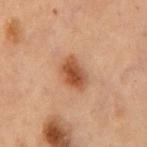Part of a total-body skin-imaging series; this lesion was reviewed on a skin check and was not flagged for biopsy.
An algorithmic analysis of the crop reported a footprint of about 7 mm² and a shape eccentricity near 0.7. And it measured a border-irregularity index near 2/10 and a color-variation rating of about 4/10. And it measured a lesion-detection confidence of about 100/100.
From the chest.
The recorded lesion diameter is about 3.5 mm.
A 15 mm crop from a total-body photograph taken for skin-cancer surveillance.
A male subject roughly 70 years of age.